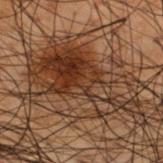{
  "biopsy_status": "not biopsied; imaged during a skin examination",
  "lighting": "cross-polarized",
  "patient": {
    "sex": "male",
    "age_approx": 50
  },
  "lesion_size": {
    "long_diameter_mm_approx": 12.5
  },
  "site": "upper back",
  "image": {
    "source": "total-body photography crop",
    "field_of_view_mm": 15
  }
}